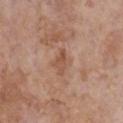follow-up = imaged on a skin check; not biopsied
lesion diameter = ~3.5 mm (longest diameter)
acquisition = ~15 mm tile from a whole-body skin photo
site = the chest
TBP lesion metrics = border irregularity of about 4.5 on a 0–10 scale, a within-lesion color-variation index near 2.5/10, and a peripheral color-asymmetry measure near 1; a nevus-likeness score of about 0/100
subject = female, in their mid- to late 80s or older